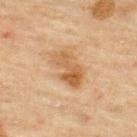follow-up — total-body-photography surveillance lesion; no biopsy | anatomic site — the upper back | tile lighting — cross-polarized | lesion size — ≈5 mm | acquisition — ~15 mm crop, total-body skin-cancer survey | patient — male, approximately 45 years of age | automated lesion analysis — a footprint of about 11 mm², an eccentricity of roughly 0.85, and a shape-asymmetry score of about 0.3 (0 = symmetric); about 9 CIELAB-L* units darker than the surrounding skin and a normalized lesion–skin contrast near 7; an automated nevus-likeness rating near 5 out of 100 and a detector confidence of about 100 out of 100 that the crop contains a lesion.